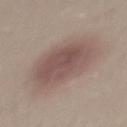Case summary:
* site · the back
* illumination · white-light illumination
* diameter · ~8 mm (longest diameter)
* image-analysis metrics · a lesion area of about 32 mm² and a shape-asymmetry score of about 0.1 (0 = symmetric); an average lesion color of about L≈52 a*≈16 b*≈21 (CIELAB), a lesion–skin lightness drop of about 10, and a normalized border contrast of about 7.5; an automated nevus-likeness rating near 95 out of 100 and lesion-presence confidence of about 100/100
* image · 15 mm crop, total-body photography
* subject · male, roughly 30 years of age